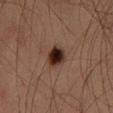{
  "biopsy_status": "not biopsied; imaged during a skin examination",
  "site": "left thigh",
  "patient": {
    "sex": "male",
    "age_approx": 35
  },
  "image": {
    "source": "total-body photography crop",
    "field_of_view_mm": 15
  },
  "lesion_size": {
    "long_diameter_mm_approx": 3.5
  },
  "automated_metrics": {
    "cielab_L": 25,
    "cielab_a": 17,
    "cielab_b": 21,
    "vs_skin_darker_L": 16.0,
    "vs_skin_contrast_norm": 15.5,
    "lesion_detection_confidence_0_100": 100
  }
}